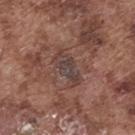Imaged with white-light lighting.
The lesion is on the upper back.
The patient is a male in their mid-70s.
A 15 mm close-up tile from a total-body photography series done for melanoma screening.
The total-body-photography lesion software estimated a footprint of about 7 mm², an outline eccentricity of about 0.9 (0 = round, 1 = elongated), and two-axis asymmetry of about 0.3.